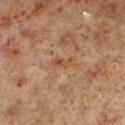On the left lower leg.
A 15 mm close-up tile from a total-body photography series done for melanoma screening.
Captured under cross-polarized illumination.
A male subject in their 60s.
Measured at roughly 3 mm in maximum diameter.